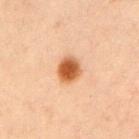A male patient approximately 55 years of age. The lesion is located on the left upper arm. Captured under cross-polarized illumination. Automated tile analysis of the lesion measured internal color variation of about 4.5 on a 0–10 scale and peripheral color asymmetry of about 1.5. A 15 mm crop from a total-body photograph taken for skin-cancer surveillance. Approximately 3 mm at its widest.